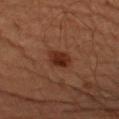biopsy_status: not biopsied; imaged during a skin examination
image:
  source: total-body photography crop
  field_of_view_mm: 15
site: right upper arm
patient:
  sex: male
  age_approx: 65
lesion_size:
  long_diameter_mm_approx: 3.0
lighting: cross-polarized